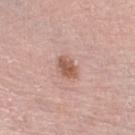<record>
  <biopsy_status>not biopsied; imaged during a skin examination</biopsy_status>
  <site>right lower leg</site>
  <automated_metrics>
    <eccentricity>0.75</eccentricity>
    <shape_asymmetry>0.3</shape_asymmetry>
    <cielab_L>57</cielab_L>
    <cielab_a>21</cielab_a>
    <cielab_b>28</cielab_b>
    <vs_skin_darker_L>11.0</vs_skin_darker_L>
    <vs_skin_contrast_norm>8.0</vs_skin_contrast_norm>
    <color_variation_0_10>3.0</color_variation_0_10>
    <peripheral_color_asymmetry>1.0</peripheral_color_asymmetry>
    <lesion_detection_confidence_0_100>100</lesion_detection_confidence_0_100>
  </automated_metrics>
  <patient>
    <sex>male</sex>
    <age_approx>70</age_approx>
  </patient>
  <lesion_size>
    <long_diameter_mm_approx>3.0</long_diameter_mm_approx>
  </lesion_size>
  <image>
    <source>total-body photography crop</source>
    <field_of_view_mm>15</field_of_view_mm>
  </image>
  <lighting>white-light</lighting>
</record>